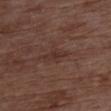This lesion was catalogued during total-body skin photography and was not selected for biopsy.
A lesion tile, about 15 mm wide, cut from a 3D total-body photograph.
The lesion is on the chest.
The patient is a female aged 78–82.